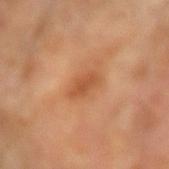No biopsy was performed on this lesion — it was imaged during a full skin examination and was not determined to be concerning. Cropped from a whole-body photographic skin survey; the tile spans about 15 mm. The total-body-photography lesion software estimated a lesion area of about 5 mm² and two-axis asymmetry of about 0.25. It also reported a border-irregularity index near 2.5/10, a within-lesion color-variation index near 1.5/10, and a peripheral color-asymmetry measure near 0.5. The analysis additionally found a classifier nevus-likeness of about 5/100. The recorded lesion diameter is about 3 mm. The lesion is located on the left forearm. The patient is a female in their mid-70s. Captured under cross-polarized illumination.